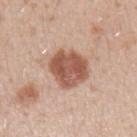Imaged during a routine full-body skin examination; the lesion was not biopsied and no histopathology is available.
Cropped from a whole-body photographic skin survey; the tile spans about 15 mm.
The tile uses white-light illumination.
The subject is a male in their 30s.
From the left upper arm.
Measured at roughly 4.5 mm in maximum diameter.
Automated image analysis of the tile measured an area of roughly 16 mm², an outline eccentricity of about 0.45 (0 = round, 1 = elongated), and a symmetry-axis asymmetry near 0.2. And it measured roughly 15 lightness units darker than nearby skin. It also reported a border-irregularity rating of about 2/10 and a within-lesion color-variation index near 4.5/10. It also reported a nevus-likeness score of about 90/100 and a detector confidence of about 100 out of 100 that the crop contains a lesion.